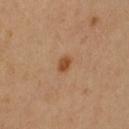Acquisition and patient details: Cropped from a total-body skin-imaging series; the visible field is about 15 mm. On the left upper arm. The total-body-photography lesion software estimated a footprint of about 3 mm², an outline eccentricity of about 0.7 (0 = round, 1 = elongated), and a symmetry-axis asymmetry near 0.25. The analysis additionally found a lesion color around L≈48 a*≈24 b*≈36 in CIELAB, about 11 CIELAB-L* units darker than the surrounding skin, and a lesion-to-skin contrast of about 8.5 (normalized; higher = more distinct). The software also gave a border-irregularity rating of about 2/10 and a peripheral color-asymmetry measure near 1. It also reported an automated nevus-likeness rating near 95 out of 100. Imaged with cross-polarized lighting. A female subject, roughly 55 years of age.